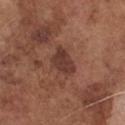Captured under white-light illumination.
Automated image analysis of the tile measured a symmetry-axis asymmetry near 0.4. It also reported a mean CIELAB color near L≈37 a*≈21 b*≈24, a lesion–skin lightness drop of about 10, and a normalized border contrast of about 8.5.
A roughly 15 mm field-of-view crop from a total-body skin photograph.
Approximately 4 mm at its widest.
From the chest.
The subject is a male approximately 75 years of age.